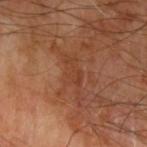Image and clinical context: The patient is a male aged 63 to 67. Automated image analysis of the tile measured border irregularity of about 7.5 on a 0–10 scale, internal color variation of about 1.5 on a 0–10 scale, and a peripheral color-asymmetry measure near 0.5. The software also gave an automated nevus-likeness rating near 0 out of 100 and lesion-presence confidence of about 90/100. The lesion is located on the left upper arm. The lesion's longest dimension is about 5 mm. This image is a 15 mm lesion crop taken from a total-body photograph. The tile uses cross-polarized illumination.